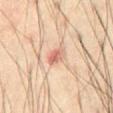patient:
  sex: male
  age_approx: 60
site: front of the torso
lighting: cross-polarized
lesion_size:
  long_diameter_mm_approx: 2.5
image:
  source: total-body photography crop
  field_of_view_mm: 15
automated_metrics:
  area_mm2_approx: 4.0
  eccentricity: 0.7
  shape_asymmetry: 0.25
  cielab_L: 59
  cielab_a: 22
  cielab_b: 28
  vs_skin_darker_L: 10.0
  vs_skin_contrast_norm: 6.5
  nevus_likeness_0_100: 20
  lesion_detection_confidence_0_100: 100